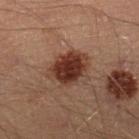biopsy_status: not biopsied; imaged during a skin examination
site: leg
patient:
  sex: male
  age_approx: 40
image:
  source: total-body photography crop
  field_of_view_mm: 15
lighting: cross-polarized